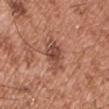<tbp_lesion>
<site>chest</site>
<lighting>white-light</lighting>
<image>
  <source>total-body photography crop</source>
  <field_of_view_mm>15</field_of_view_mm>
</image>
<automated_metrics>
  <area_mm2_approx>9.0</area_mm2_approx>
  <eccentricity>0.75</eccentricity>
  <shape_asymmetry>0.2</shape_asymmetry>
</automated_metrics>
<patient>
  <sex>male</sex>
  <age_approx>55</age_approx>
</patient>
</tbp_lesion>